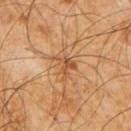Automated tile analysis of the lesion measured border irregularity of about 8 on a 0–10 scale. A male patient roughly 60 years of age. A 15 mm crop from a total-body photograph taken for skin-cancer surveillance. From the right upper arm. About 3 mm across.